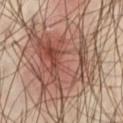Notes:
- workup · catalogued during a skin exam; not biopsied
- lesion size · about 10 mm
- anatomic site · the left thigh
- image-analysis metrics · an area of roughly 49 mm², a shape eccentricity near 0.55, and a symmetry-axis asymmetry near 0.4; a mean CIELAB color near L≈52 a*≈21 b*≈26 and a normalized border contrast of about 7.5; a border-irregularity index near 8/10 and internal color variation of about 9 on a 0–10 scale; an automated nevus-likeness rating near 50 out of 100 and a lesion-detection confidence of about 95/100
- patient · male, roughly 40 years of age
- tile lighting · cross-polarized
- image source · total-body-photography crop, ~15 mm field of view